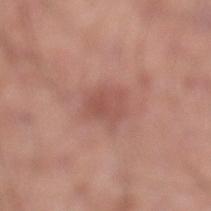{"biopsy_status": "not biopsied; imaged during a skin examination", "site": "leg", "patient": {"sex": "male", "age_approx": 20}, "image": {"source": "total-body photography crop", "field_of_view_mm": 15}, "lighting": "white-light", "automated_metrics": {"area_mm2_approx": 5.5, "vs_skin_darker_L": 7.0, "vs_skin_contrast_norm": 5.5, "border_irregularity_0_10": 2.0, "peripheral_color_asymmetry": 1.0}}